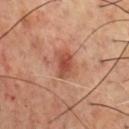Case summary:
- diameter — ≈3.5 mm
- location — the chest
- image — ~15 mm crop, total-body skin-cancer survey
- TBP lesion metrics — a classifier nevus-likeness of about 25/100
- tile lighting — cross-polarized illumination
- subject — male, aged approximately 70